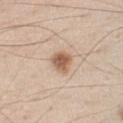The lesion was photographed on a routine skin check and not biopsied; there is no pathology result. The patient is a male approximately 45 years of age. A roughly 15 mm field-of-view crop from a total-body skin photograph. From the chest.